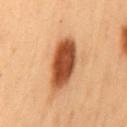This lesion was catalogued during total-body skin photography and was not selected for biopsy. A 15 mm close-up extracted from a 3D total-body photography capture. The recorded lesion diameter is about 6 mm. The total-body-photography lesion software estimated a footprint of about 15 mm² and an outline eccentricity of about 0.8 (0 = round, 1 = elongated). A male subject, aged 53–57. From the mid back.